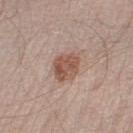The lesion was photographed on a routine skin check and not biopsied; there is no pathology result.
On the left lower leg.
A 15 mm close-up extracted from a 3D total-body photography capture.
Approximately 4 mm at its widest.
The subject is a male aged 53 to 57.
An algorithmic analysis of the crop reported roughly 11 lightness units darker than nearby skin and a normalized border contrast of about 8. The software also gave a border-irregularity rating of about 2/10, a within-lesion color-variation index near 4/10, and a peripheral color-asymmetry measure near 1.5.